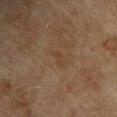workup: no biopsy performed (imaged during a skin exam) | subject: male, aged approximately 75 | size: ~2.5 mm (longest diameter) | automated lesion analysis: a lesion–skin lightness drop of about 4 and a normalized border contrast of about 4.5; border irregularity of about 5 on a 0–10 scale and internal color variation of about 0 on a 0–10 scale | acquisition: total-body-photography crop, ~15 mm field of view | location: the left upper arm.